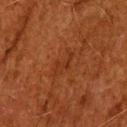| field | value |
|---|---|
| location | the head or neck |
| image | ~15 mm crop, total-body skin-cancer survey |
| lighting | cross-polarized |
| lesion diameter | about 3.5 mm |
| patient | male, in their 80s |